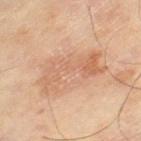Case summary:
- follow-up · total-body-photography surveillance lesion; no biopsy
- image source · ~15 mm crop, total-body skin-cancer survey
- site · the left thigh
- subject · male, aged around 65
- lighting · cross-polarized